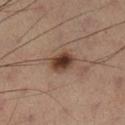The recorded lesion diameter is about 3.5 mm. The tile uses cross-polarized illumination. The patient is a male aged around 65. A 15 mm close-up tile from a total-body photography series done for melanoma screening. An algorithmic analysis of the crop reported a nevus-likeness score of about 95/100 and a detector confidence of about 100 out of 100 that the crop contains a lesion. The lesion is on the left lower leg.